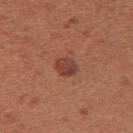Acquisition and patient details: This is a white-light tile. A female subject in their mid- to late 30s. The total-body-photography lesion software estimated an area of roughly 5 mm² and an outline eccentricity of about 0.5 (0 = round, 1 = elongated). The analysis additionally found an average lesion color of about L≈42 a*≈25 b*≈28 (CIELAB), a lesion–skin lightness drop of about 10, and a normalized border contrast of about 8. The software also gave a border-irregularity rating of about 1.5/10. And it measured a classifier nevus-likeness of about 70/100 and a detector confidence of about 100 out of 100 that the crop contains a lesion. Measured at roughly 2.5 mm in maximum diameter. The lesion is located on the right upper arm. A roughly 15 mm field-of-view crop from a total-body skin photograph.